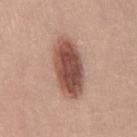The lesion was photographed on a routine skin check and not biopsied; there is no pathology result. Measured at roughly 7 mm in maximum diameter. A female patient, aged 23 to 27. This is a white-light tile. A 15 mm close-up extracted from a 3D total-body photography capture. Located on the right thigh.